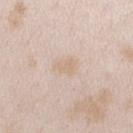workup: imaged on a skin check; not biopsied
site: the arm
subject: female, aged approximately 25
lesion diameter: about 2.5 mm
illumination: white-light illumination
image source: total-body-photography crop, ~15 mm field of view